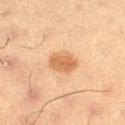{
  "biopsy_status": "not biopsied; imaged during a skin examination",
  "patient": {
    "sex": "male",
    "age_approx": 55
  },
  "image": {
    "source": "total-body photography crop",
    "field_of_view_mm": 15
  },
  "lesion_size": {
    "long_diameter_mm_approx": 3.5
  },
  "site": "leg"
}